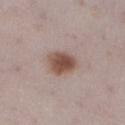This lesion was catalogued during total-body skin photography and was not selected for biopsy. This is a white-light tile. The lesion is on the leg. Cropped from a whole-body photographic skin survey; the tile spans about 15 mm. A female subject, in their 30s.